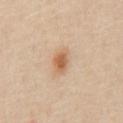Captured during whole-body skin photography for melanoma surveillance; the lesion was not biopsied. Longest diameter approximately 3.5 mm. The lesion is on the abdomen. Cropped from a total-body skin-imaging series; the visible field is about 15 mm. The subject is a male in their mid-60s.